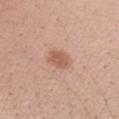| field | value |
|---|---|
| notes | total-body-photography surveillance lesion; no biopsy |
| tile lighting | white-light illumination |
| location | the right upper arm |
| lesion diameter | ~3 mm (longest diameter) |
| image source | 15 mm crop, total-body photography |
| patient | female, approximately 40 years of age |